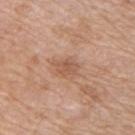Captured during whole-body skin photography for melanoma surveillance; the lesion was not biopsied.
Automated image analysis of the tile measured a border-irregularity index near 2/10, a within-lesion color-variation index near 2.5/10, and peripheral color asymmetry of about 1. And it measured a classifier nevus-likeness of about 0/100.
The lesion is located on the upper back.
The patient is a female about 40 years old.
A lesion tile, about 15 mm wide, cut from a 3D total-body photograph.
Approximately 2.5 mm at its widest.
Captured under white-light illumination.